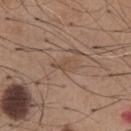Impression:
Captured during whole-body skin photography for melanoma surveillance; the lesion was not biopsied.
Context:
A 15 mm close-up extracted from a 3D total-body photography capture. Automated tile analysis of the lesion measured a footprint of about 3 mm². And it measured an average lesion color of about L≈49 a*≈17 b*≈28 (CIELAB) and roughly 6 lightness units darker than nearby skin. And it measured a nevus-likeness score of about 0/100. A male subject roughly 65 years of age. Captured under white-light illumination. On the chest.